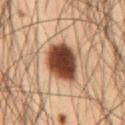biopsy status: total-body-photography surveillance lesion; no biopsy | body site: the abdomen | image source: ~15 mm crop, total-body skin-cancer survey | illumination: cross-polarized | TBP lesion metrics: an area of roughly 15 mm², a shape eccentricity near 0.7, and a shape-asymmetry score of about 0.15 (0 = symmetric); an average lesion color of about L≈35 a*≈19 b*≈26 (CIELAB), roughly 19 lightness units darker than nearby skin, and a normalized lesion–skin contrast near 15.5; border irregularity of about 2 on a 0–10 scale, a within-lesion color-variation index near 6/10, and peripheral color asymmetry of about 1.5; an automated nevus-likeness rating near 100 out of 100 and lesion-presence confidence of about 100/100 | patient: male, aged around 55 | lesion diameter: about 5 mm.